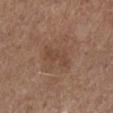Recorded during total-body skin imaging; not selected for excision or biopsy.
Longest diameter approximately 4 mm.
Automated image analysis of the tile measured border irregularity of about 5.5 on a 0–10 scale, a within-lesion color-variation index near 1/10, and peripheral color asymmetry of about 0.5. It also reported an automated nevus-likeness rating near 0 out of 100.
The lesion is on the left lower leg.
A 15 mm close-up tile from a total-body photography series done for melanoma screening.
This is a white-light tile.
The subject is a male aged 73 to 77.